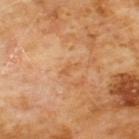Background:
Automated tile analysis of the lesion measured a footprint of about 2 mm² and a shape-asymmetry score of about 0.5 (0 = symmetric). The lesion's longest dimension is about 2.5 mm. The tile uses cross-polarized illumination. Located on the chest. This image is a 15 mm lesion crop taken from a total-body photograph. The patient is a male about 60 years old.